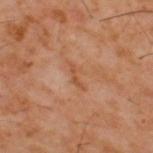{
  "biopsy_status": "not biopsied; imaged during a skin examination",
  "automated_metrics": {
    "area_mm2_approx": 3.0,
    "shape_asymmetry": 0.6,
    "cielab_L": 48,
    "cielab_a": 22,
    "cielab_b": 33,
    "vs_skin_darker_L": 6.0,
    "vs_skin_contrast_norm": 5.5,
    "border_irregularity_0_10": 7.0,
    "color_variation_0_10": 0.0,
    "nevus_likeness_0_100": 0,
    "lesion_detection_confidence_0_100": 95
  },
  "patient": {
    "sex": "male",
    "age_approx": 60
  },
  "image": {
    "source": "total-body photography crop",
    "field_of_view_mm": 15
  },
  "site": "upper back"
}